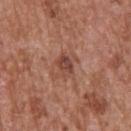Clinical impression:
Recorded during total-body skin imaging; not selected for excision or biopsy.
Image and clinical context:
A male subject, about 65 years old. A 15 mm close-up extracted from a 3D total-body photography capture. Located on the back. Captured under white-light illumination. Longest diameter approximately 2.5 mm. Automated tile analysis of the lesion measured a footprint of about 4 mm², a shape eccentricity near 0.7, and a symmetry-axis asymmetry near 0.2. The analysis additionally found a lesion color around L≈44 a*≈23 b*≈27 in CIELAB, about 10 CIELAB-L* units darker than the surrounding skin, and a normalized border contrast of about 7.5.